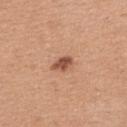Context:
The tile uses white-light illumination. Longest diameter approximately 2.5 mm. Located on the upper back. The total-body-photography lesion software estimated a footprint of about 4 mm² and a shape eccentricity near 0.8. And it measured border irregularity of about 1.5 on a 0–10 scale, a within-lesion color-variation index near 2/10, and a peripheral color-asymmetry measure near 1. The patient is a female aged 28 to 32. A lesion tile, about 15 mm wide, cut from a 3D total-body photograph.